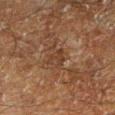Findings:
- workup — total-body-photography surveillance lesion; no biopsy
- tile lighting — cross-polarized
- subject — male, about 60 years old
- image source — total-body-photography crop, ~15 mm field of view
- anatomic site — the left lower leg
- size — ~2.5 mm (longest diameter)
- automated lesion analysis — a footprint of about 3.5 mm², a shape eccentricity near 0.7, and a symmetry-axis asymmetry near 0.55; border irregularity of about 6.5 on a 0–10 scale; a classifier nevus-likeness of about 0/100 and lesion-presence confidence of about 80/100